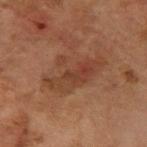This lesion was catalogued during total-body skin photography and was not selected for biopsy. Located on the arm. A female patient, aged around 60. The total-body-photography lesion software estimated a within-lesion color-variation index near 5.5/10 and a peripheral color-asymmetry measure near 2. The software also gave a nevus-likeness score of about 5/100 and a detector confidence of about 100 out of 100 that the crop contains a lesion. The lesion's longest dimension is about 7 mm. A 15 mm crop from a total-body photograph taken for skin-cancer surveillance. This is a cross-polarized tile.